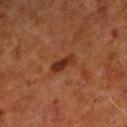Clinical impression:
Recorded during total-body skin imaging; not selected for excision or biopsy.
Clinical summary:
A 15 mm close-up tile from a total-body photography series done for melanoma screening. The subject is a male about 70 years old. The lesion is on the right upper arm. Measured at roughly 3 mm in maximum diameter.